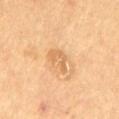Clinical impression: No biopsy was performed on this lesion — it was imaged during a full skin examination and was not determined to be concerning. Acquisition and patient details: The lesion is located on the leg. Imaged with cross-polarized lighting. The subject is a male aged around 60. The recorded lesion diameter is about 3 mm. A 15 mm close-up tile from a total-body photography series done for melanoma screening.